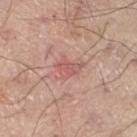Notes:
– notes · imaged on a skin check; not biopsied
– lesion size · ≈3.5 mm
– TBP lesion metrics · a lesion area of about 8 mm², a shape eccentricity near 0.65, and two-axis asymmetry of about 0.3; a mean CIELAB color near L≈59 a*≈23 b*≈24, roughly 7 lightness units darker than nearby skin, and a lesion-to-skin contrast of about 5 (normalized; higher = more distinct); a border-irregularity rating of about 3.5/10; an automated nevus-likeness rating near 0 out of 100 and lesion-presence confidence of about 100/100
– acquisition · 15 mm crop, total-body photography
– location · the right thigh
– subject · male, approximately 70 years of age